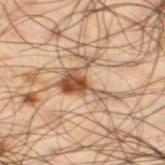– workup: no biopsy performed (imaged during a skin exam)
– TBP lesion metrics: a footprint of about 10 mm², a shape eccentricity near 0.65, and two-axis asymmetry of about 0.7; an average lesion color of about L≈41 a*≈15 b*≈26 (CIELAB), about 11 CIELAB-L* units darker than the surrounding skin, and a normalized border contrast of about 9; a border-irregularity index near 9.5/10, a color-variation rating of about 6/10, and radial color variation of about 2
– lesion size: ≈5.5 mm
– illumination: cross-polarized illumination
– patient: male, about 50 years old
– site: the left thigh
– image: total-body-photography crop, ~15 mm field of view